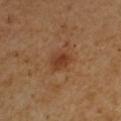Imaged during a routine full-body skin examination; the lesion was not biopsied and no histopathology is available. A male subject about 50 years old. A 15 mm crop from a total-body photograph taken for skin-cancer surveillance. The lesion is located on the right upper arm. Automated tile analysis of the lesion measured a mean CIELAB color near L≈38 a*≈22 b*≈33 and a normalized lesion–skin contrast near 7.5. And it measured a border-irregularity rating of about 2/10, internal color variation of about 2.5 on a 0–10 scale, and radial color variation of about 1. Measured at roughly 3 mm in maximum diameter.